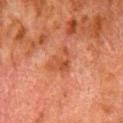This lesion was catalogued during total-body skin photography and was not selected for biopsy. On the right lower leg. A male patient roughly 80 years of age. A 15 mm crop from a total-body photograph taken for skin-cancer surveillance. About 3.5 mm across.